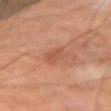Clinical impression: No biopsy was performed on this lesion — it was imaged during a full skin examination and was not determined to be concerning. Clinical summary: From the right upper arm. The subject is a male aged approximately 60. Approximately 3 mm at its widest. This image is a 15 mm lesion crop taken from a total-body photograph.